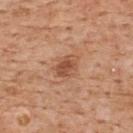biopsy status: total-body-photography surveillance lesion; no biopsy
lesion diameter: about 3 mm
image: ~15 mm tile from a whole-body skin photo
patient: male, aged around 60
tile lighting: white-light
automated metrics: a footprint of about 5.5 mm², an eccentricity of roughly 0.65, and two-axis asymmetry of about 0.25; a nevus-likeness score of about 75/100 and a detector confidence of about 100 out of 100 that the crop contains a lesion
location: the upper back